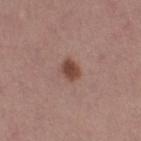| key | value |
|---|---|
| workup | imaged on a skin check; not biopsied |
| body site | the right thigh |
| automated lesion analysis | an eccentricity of roughly 0.65 and a symmetry-axis asymmetry near 0.2; a lesion color around L≈45 a*≈21 b*≈25 in CIELAB, roughly 12 lightness units darker than nearby skin, and a normalized lesion–skin contrast near 9; a within-lesion color-variation index near 2.5/10 and peripheral color asymmetry of about 1; an automated nevus-likeness rating near 100 out of 100 and lesion-presence confidence of about 100/100 |
| image | 15 mm crop, total-body photography |
| patient | female, about 50 years old |